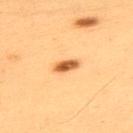The lesion was photographed on a routine skin check and not biopsied; there is no pathology result.
Located on the back.
Longest diameter approximately 3 mm.
This is a cross-polarized tile.
Cropped from a total-body skin-imaging series; the visible field is about 15 mm.
A male subject, approximately 55 years of age.